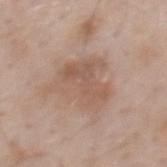Imaged during a routine full-body skin examination; the lesion was not biopsied and no histopathology is available. This image is a 15 mm lesion crop taken from a total-body photograph. A male patient aged approximately 60. Located on the mid back. The tile uses white-light illumination.